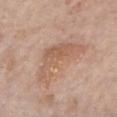tile lighting: white-light illumination
automated metrics: border irregularity of about 7.5 on a 0–10 scale and peripheral color asymmetry of about 1
image: total-body-photography crop, ~15 mm field of view
lesion size: about 7.5 mm
subject: female, in their mid- to late 80s or older
body site: the chest